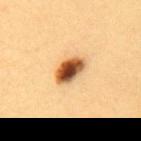follow-up — no biopsy performed (imaged during a skin exam) | patient — female, aged approximately 30 | lighting — cross-polarized illumination | image-analysis metrics — a border-irregularity index near 2/10 and internal color variation of about 9 on a 0–10 scale; an automated nevus-likeness rating near 100 out of 100 and a detector confidence of about 100 out of 100 that the crop contains a lesion | imaging modality — 15 mm crop, total-body photography | body site — the upper back.